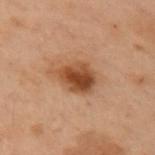| feature | finding |
|---|---|
| biopsy status | total-body-photography surveillance lesion; no biopsy |
| site | the left arm |
| TBP lesion metrics | a mean CIELAB color near L≈40 a*≈20 b*≈30 and a lesion–skin lightness drop of about 11; a border-irregularity index near 2.5/10 and a within-lesion color-variation index near 6/10 |
| size | ≈4 mm |
| illumination | cross-polarized |
| imaging modality | ~15 mm tile from a whole-body skin photo |
| patient | female, approximately 55 years of age |